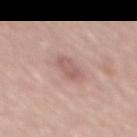image:
  source: total-body photography crop
  field_of_view_mm: 15
patient:
  sex: male
  age_approx: 60
site: mid back